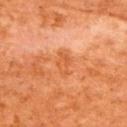{
  "biopsy_status": "not biopsied; imaged during a skin examination",
  "image": {
    "source": "total-body photography crop",
    "field_of_view_mm": 15
  },
  "lesion_size": {
    "long_diameter_mm_approx": 3.0
  },
  "site": "upper back",
  "patient": {
    "sex": "male",
    "age_approx": 65
  },
  "automated_metrics": {
    "area_mm2_approx": 4.0,
    "shape_asymmetry": 0.3,
    "border_irregularity_0_10": 3.5,
    "color_variation_0_10": 2.0
  },
  "lighting": "cross-polarized"
}